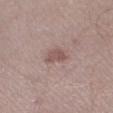Assessment:
This lesion was catalogued during total-body skin photography and was not selected for biopsy.
Clinical summary:
A roughly 15 mm field-of-view crop from a total-body skin photograph. This is a white-light tile. About 2.5 mm across. Located on the left lower leg. The subject is a male approximately 40 years of age.